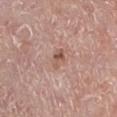Case summary:
– workup: catalogued during a skin exam; not biopsied
– automated lesion analysis: a border-irregularity index near 2.5/10 and radial color variation of about 1; a lesion-detection confidence of about 100/100
– patient: male, about 75 years old
– diameter: about 2.5 mm
– imaging modality: ~15 mm crop, total-body skin-cancer survey
– illumination: white-light illumination
– body site: the left lower leg